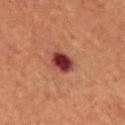Clinical impression:
Imaged during a routine full-body skin examination; the lesion was not biopsied and no histopathology is available.
Acquisition and patient details:
Captured under cross-polarized illumination. The lesion is on the chest. Longest diameter approximately 3 mm. A region of skin cropped from a whole-body photographic capture, roughly 15 mm wide. A male patient aged approximately 65.